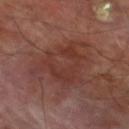follow-up: no biopsy performed (imaged during a skin exam)
size: ~6.5 mm (longest diameter)
acquisition: ~15 mm crop, total-body skin-cancer survey
patient: male, aged around 70
site: the left lower leg
illumination: cross-polarized illumination